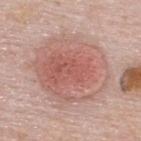A female subject, aged approximately 50. The lesion is located on the upper back. Cropped from a whole-body photographic skin survey; the tile spans about 15 mm.